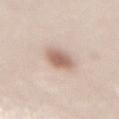Clinical impression: Recorded during total-body skin imaging; not selected for excision or biopsy. Clinical summary: Measured at roughly 3.5 mm in maximum diameter. A female subject, aged around 25. A 15 mm crop from a total-body photograph taken for skin-cancer surveillance. The tile uses white-light illumination. The lesion is on the mid back.